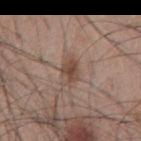follow-up: imaged on a skin check; not biopsied.